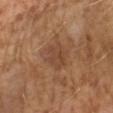notes: total-body-photography surveillance lesion; no biopsy
imaging modality: 15 mm crop, total-body photography
tile lighting: cross-polarized illumination
image-analysis metrics: an eccentricity of roughly 0.65 and two-axis asymmetry of about 0.2; a mean CIELAB color near L≈40 a*≈18 b*≈28, a lesion–skin lightness drop of about 6, and a normalized lesion–skin contrast near 5.5
subject: male, about 60 years old
site: the left forearm
diameter: ~3.5 mm (longest diameter)